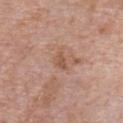This lesion was catalogued during total-body skin photography and was not selected for biopsy.
The patient is a male aged 58–62.
The tile uses white-light illumination.
A 15 mm close-up extracted from a 3D total-body photography capture.
The lesion is on the chest.
About 2.5 mm across.